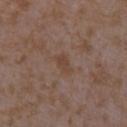No biopsy was performed on this lesion — it was imaged during a full skin examination and was not determined to be concerning.
The lesion-visualizer software estimated a footprint of about 3.5 mm² and an outline eccentricity of about 0.85 (0 = round, 1 = elongated). The software also gave a mean CIELAB color near L≈42 a*≈17 b*≈26 and a lesion-to-skin contrast of about 6 (normalized; higher = more distinct). It also reported an automated nevus-likeness rating near 0 out of 100.
Captured under white-light illumination.
About 2.5 mm across.
The lesion is on the right forearm.
A 15 mm close-up extracted from a 3D total-body photography capture.
A female patient approximately 35 years of age.